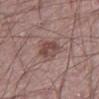Q: Is there a histopathology result?
A: total-body-photography surveillance lesion; no biopsy
Q: What lighting was used for the tile?
A: white-light
Q: Patient demographics?
A: male, aged 18 to 22
Q: Lesion location?
A: the left thigh
Q: What kind of image is this?
A: ~15 mm tile from a whole-body skin photo
Q: Automated lesion metrics?
A: a mean CIELAB color near L≈46 a*≈19 b*≈20, roughly 9 lightness units darker than nearby skin, and a normalized border contrast of about 7.5; border irregularity of about 2.5 on a 0–10 scale, a within-lesion color-variation index near 4.5/10, and peripheral color asymmetry of about 2; an automated nevus-likeness rating near 25 out of 100 and a detector confidence of about 100 out of 100 that the crop contains a lesion